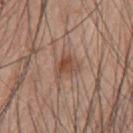Recorded during total-body skin imaging; not selected for excision or biopsy.
This image is a 15 mm lesion crop taken from a total-body photograph.
The patient is a male approximately 60 years of age.
The lesion is on the chest.